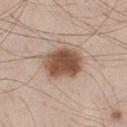notes = total-body-photography surveillance lesion; no biopsy | lighting = white-light illumination | body site = the left thigh | size = about 5 mm | subject = male, aged around 45 | imaging modality = 15 mm crop, total-body photography.